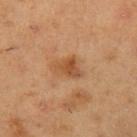Context: The tile uses cross-polarized illumination. A male patient, about 55 years old. On the left upper arm. A lesion tile, about 15 mm wide, cut from a 3D total-body photograph. Measured at roughly 3.5 mm in maximum diameter.